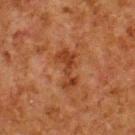Clinical impression:
The lesion was tiled from a total-body skin photograph and was not biopsied.
Context:
The lesion is on the upper back. About 4.5 mm across. The subject is a male in their 80s. A roughly 15 mm field-of-view crop from a total-body skin photograph. The tile uses cross-polarized illumination.